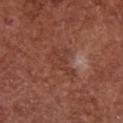* follow-up — no biopsy performed (imaged during a skin exam)
* diameter — about 5 mm
* patient — female, roughly 75 years of age
* location — the chest
* image source — ~15 mm tile from a whole-body skin photo